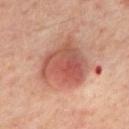This lesion was catalogued during total-body skin photography and was not selected for biopsy. Cropped from a total-body skin-imaging series; the visible field is about 15 mm. Imaged with cross-polarized lighting. The lesion is on the mid back. A male subject, about 65 years old.